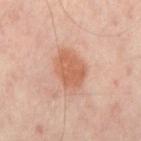Located on the mid back. A patient in their mid-50s. Cropped from a whole-body photographic skin survey; the tile spans about 15 mm.